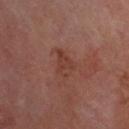Clinical impression:
Imaged during a routine full-body skin examination; the lesion was not biopsied and no histopathology is available.
Clinical summary:
Cropped from a total-body skin-imaging series; the visible field is about 15 mm. Measured at roughly 3 mm in maximum diameter. Automated tile analysis of the lesion measured a lesion area of about 5 mm². The analysis additionally found a border-irregularity rating of about 3/10. The patient is a male about 60 years old. The lesion is on the head or neck. This is a cross-polarized tile.